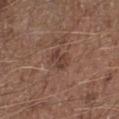Impression: Captured during whole-body skin photography for melanoma surveillance; the lesion was not biopsied. Clinical summary: Automated tile analysis of the lesion measured a border-irregularity index near 3/10 and radial color variation of about 1. The lesion's longest dimension is about 2.5 mm. A roughly 15 mm field-of-view crop from a total-body skin photograph. Imaged with white-light lighting. A male subject aged 73–77. On the left lower leg.